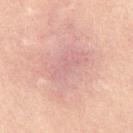The lesion was photographed on a routine skin check and not biopsied; there is no pathology result.
From the chest.
The tile uses cross-polarized illumination.
Measured at roughly 7.5 mm in maximum diameter.
A 15 mm close-up extracted from a 3D total-body photography capture.
The patient is a male in their 30s.
The lesion-visualizer software estimated a mean CIELAB color near L≈55 a*≈18 b*≈20, roughly 5 lightness units darker than nearby skin, and a normalized lesion–skin contrast near 4.5.